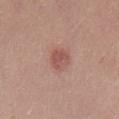{"biopsy_status": "not biopsied; imaged during a skin examination", "image": {"source": "total-body photography crop", "field_of_view_mm": 15}, "lighting": "white-light", "site": "chest", "automated_metrics": {"vs_skin_darker_L": 9.0, "vs_skin_contrast_norm": 6.5, "border_irregularity_0_10": 1.5, "color_variation_0_10": 3.5, "peripheral_color_asymmetry": 1.0, "nevus_likeness_0_100": 70, "lesion_detection_confidence_0_100": 100}, "patient": {"sex": "male", "age_approx": 25}}